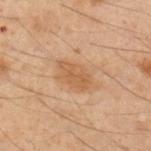follow-up=imaged on a skin check; not biopsied
diameter=≈4 mm
image-analysis metrics=a lesion area of about 7 mm²; a border-irregularity index near 2.5/10, a color-variation rating of about 1.5/10, and peripheral color asymmetry of about 0.5
body site=the right upper arm
illumination=cross-polarized
image=~15 mm crop, total-body skin-cancer survey
patient=male, aged approximately 50